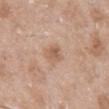Recorded during total-body skin imaging; not selected for excision or biopsy.
On the back.
A male subject about 50 years old.
This is a white-light tile.
An algorithmic analysis of the crop reported a lesion area of about 4.5 mm², an outline eccentricity of about 0.65 (0 = round, 1 = elongated), and a symmetry-axis asymmetry near 0.3. And it measured an average lesion color of about L≈59 a*≈19 b*≈30 (CIELAB) and roughly 9 lightness units darker than nearby skin.
Cropped from a total-body skin-imaging series; the visible field is about 15 mm.